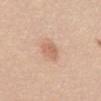Q: Was this lesion biopsied?
A: no biopsy performed (imaged during a skin exam)
Q: Lesion size?
A: ≈2.5 mm
Q: Where on the body is the lesion?
A: the abdomen
Q: Who is the patient?
A: female, roughly 55 years of age
Q: What is the imaging modality?
A: ~15 mm crop, total-body skin-cancer survey